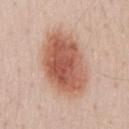Q: Is there a histopathology result?
A: catalogued during a skin exam; not biopsied
Q: What is the lesion's diameter?
A: ≈8 mm
Q: What is the imaging modality?
A: 15 mm crop, total-body photography
Q: Automated lesion metrics?
A: an outline eccentricity of about 0.8 (0 = round, 1 = elongated) and a shape-asymmetry score of about 0.1 (0 = symmetric); a border-irregularity index near 1.5/10, internal color variation of about 6.5 on a 0–10 scale, and peripheral color asymmetry of about 2; a classifier nevus-likeness of about 100/100 and a detector confidence of about 100 out of 100 that the crop contains a lesion
Q: What are the patient's age and sex?
A: male, aged approximately 30
Q: What lighting was used for the tile?
A: white-light
Q: Where on the body is the lesion?
A: the chest